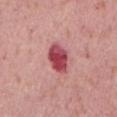The lesion was tiled from a total-body skin photograph and was not biopsied.
Approximately 4 mm at its widest.
A roughly 15 mm field-of-view crop from a total-body skin photograph.
A male patient aged approximately 70.
From the back.
Automated image analysis of the tile measured a shape eccentricity near 0.75. And it measured border irregularity of about 2 on a 0–10 scale and peripheral color asymmetry of about 1.5. It also reported a nevus-likeness score of about 0/100 and a lesion-detection confidence of about 100/100.